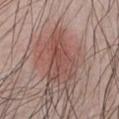No biopsy was performed on this lesion — it was imaged during a full skin examination and was not determined to be concerning.
The patient is a male in their mid- to late 20s.
A roughly 15 mm field-of-view crop from a total-body skin photograph.
This is a white-light tile.
The lesion is on the chest.
Longest diameter approximately 6.5 mm.